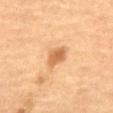biopsy status: catalogued during a skin exam; not biopsied
size: ~3 mm (longest diameter)
image: 15 mm crop, total-body photography
patient: female, approximately 70 years of age
site: the mid back
lighting: cross-polarized